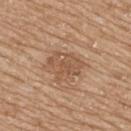notes = catalogued during a skin exam; not biopsied | lighting = white-light illumination | patient = female, aged 68–72 | imaging modality = ~15 mm crop, total-body skin-cancer survey | size = about 4.5 mm | location = the upper back.